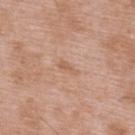{"biopsy_status": "not biopsied; imaged during a skin examination", "patient": {"sex": "male", "age_approx": 50}, "image": {"source": "total-body photography crop", "field_of_view_mm": 15}, "site": "upper back"}